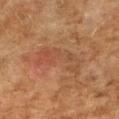<case>
  <image>
    <source>total-body photography crop</source>
    <field_of_view_mm>15</field_of_view_mm>
  </image>
  <automated_metrics>
    <cielab_L>43</cielab_L>
    <cielab_a>21</cielab_a>
    <cielab_b>30</cielab_b>
    <vs_skin_darker_L>5.0</vs_skin_darker_L>
    <border_irregularity_0_10>7.5</border_irregularity_0_10>
    <color_variation_0_10>6.0</color_variation_0_10>
    <peripheral_color_asymmetry>2.5</peripheral_color_asymmetry>
  </automated_metrics>
  <patient>
    <sex>female</sex>
    <age_approx>60</age_approx>
  </patient>
  <site>right upper arm</site>
  <lighting>cross-polarized</lighting>
  <lesion_size>
    <long_diameter_mm_approx>6.0</long_diameter_mm_approx>
  </lesion_size>
</case>